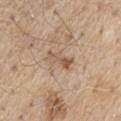Clinical impression: The lesion was photographed on a routine skin check and not biopsied; there is no pathology result. Image and clinical context: Captured under white-light illumination. A male patient, roughly 70 years of age. The recorded lesion diameter is about 3.5 mm. The lesion-visualizer software estimated a shape-asymmetry score of about 0.3 (0 = symmetric). And it measured a classifier nevus-likeness of about 0/100. A region of skin cropped from a whole-body photographic capture, roughly 15 mm wide. The lesion is located on the chest.